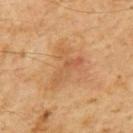Imaged during a routine full-body skin examination; the lesion was not biopsied and no histopathology is available. Cropped from a whole-body photographic skin survey; the tile spans about 15 mm. A male patient, aged approximately 60. From the mid back. Approximately 5 mm at its widest. Captured under cross-polarized illumination. An algorithmic analysis of the crop reported a lesion area of about 9 mm² and a shape-asymmetry score of about 0.65 (0 = symmetric). The analysis additionally found a mean CIELAB color near L≈52 a*≈20 b*≈36, roughly 7 lightness units darker than nearby skin, and a normalized border contrast of about 5. It also reported a color-variation rating of about 5.5/10 and peripheral color asymmetry of about 2. And it measured an automated nevus-likeness rating near 0 out of 100 and lesion-presence confidence of about 100/100.